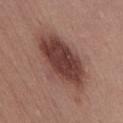Findings:
– follow-up · imaged on a skin check; not biopsied
– site · the abdomen
– acquisition · total-body-photography crop, ~15 mm field of view
– subject · female, about 50 years old